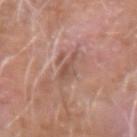Part of a total-body skin-imaging series; this lesion was reviewed on a skin check and was not flagged for biopsy.
A male patient aged approximately 75.
The lesion is located on the right forearm.
A lesion tile, about 15 mm wide, cut from a 3D total-body photograph.
Imaged with white-light lighting.
About 3 mm across.